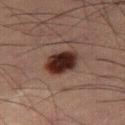Impression:
Recorded during total-body skin imaging; not selected for excision or biopsy.
Acquisition and patient details:
A close-up tile cropped from a whole-body skin photograph, about 15 mm across. A male patient approximately 55 years of age. Imaged with cross-polarized lighting. On the left thigh. The lesion's longest dimension is about 4.5 mm. The lesion-visualizer software estimated a lesion color around L≈24 a*≈17 b*≈20 in CIELAB, a lesion–skin lightness drop of about 16, and a normalized lesion–skin contrast near 15.5. The analysis additionally found border irregularity of about 1.5 on a 0–10 scale and radial color variation of about 1.5. And it measured a nevus-likeness score of about 100/100 and lesion-presence confidence of about 100/100.